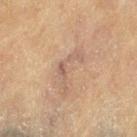Assessment:
No biopsy was performed on this lesion — it was imaged during a full skin examination and was not determined to be concerning.
Image and clinical context:
A female patient, about 80 years old. Imaged with cross-polarized lighting. The lesion's longest dimension is about 5.5 mm. A close-up tile cropped from a whole-body skin photograph, about 15 mm across. The lesion is on the leg.